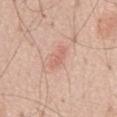follow-up — no biopsy performed (imaged during a skin exam)
automated metrics — a lesion area of about 4 mm² and a shape eccentricity near 0.85; an average lesion color of about L≈64 a*≈24 b*≈28 (CIELAB), roughly 7 lightness units darker than nearby skin, and a normalized border contrast of about 5; an automated nevus-likeness rating near 15 out of 100 and a detector confidence of about 100 out of 100 that the crop contains a lesion
patient — male, in their 70s
lesion size — ≈3 mm
location — the abdomen
image source — 15 mm crop, total-body photography